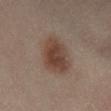biopsy status: total-body-photography surveillance lesion; no biopsy
image: ~15 mm crop, total-body skin-cancer survey
diameter: ≈5.5 mm
location: the left leg
automated metrics: a lesion color around L≈43 a*≈17 b*≈24 in CIELAB; a border-irregularity index near 2/10 and a within-lesion color-variation index near 4/10
patient: female, aged approximately 40
tile lighting: cross-polarized illumination